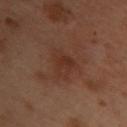biopsy status: no biopsy performed (imaged during a skin exam)
subject: female, in their mid- to late 50s
tile lighting: cross-polarized illumination
anatomic site: the chest
image: ~15 mm tile from a whole-body skin photo